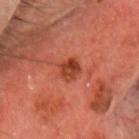Findings:
• lesion diameter: ~2.5 mm (longest diameter)
• automated lesion analysis: a nevus-likeness score of about 90/100 and lesion-presence confidence of about 100/100
• site: the head or neck
• patient: male, aged 68–72
• acquisition: ~15 mm crop, total-body skin-cancer survey